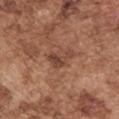Q: Is there a histopathology result?
A: no biopsy performed (imaged during a skin exam)
Q: How was the tile lit?
A: white-light
Q: Lesion location?
A: the left upper arm
Q: What is the lesion's diameter?
A: ≈3.5 mm
Q: How was this image acquired?
A: ~15 mm crop, total-body skin-cancer survey
Q: What are the patient's age and sex?
A: male, aged 73–77